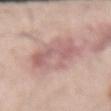Clinical impression: No biopsy was performed on this lesion — it was imaged during a full skin examination and was not determined to be concerning. Acquisition and patient details: Cropped from a whole-body photographic skin survey; the tile spans about 15 mm. Measured at roughly 6 mm in maximum diameter. The subject is a male about 60 years old. From the right forearm. This is a white-light tile.